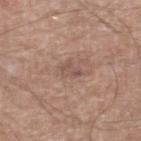The lesion was photographed on a routine skin check and not biopsied; there is no pathology result.
A male patient, in their mid- to late 60s.
A 15 mm close-up tile from a total-body photography series done for melanoma screening.
From the right lower leg.